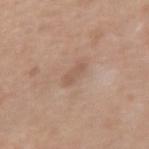Part of a total-body skin-imaging series; this lesion was reviewed on a skin check and was not flagged for biopsy. On the right forearm. A roughly 15 mm field-of-view crop from a total-body skin photograph. The recorded lesion diameter is about 2.5 mm. Captured under white-light illumination. A female patient, about 50 years old.